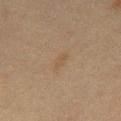Captured during whole-body skin photography for melanoma surveillance; the lesion was not biopsied.
A male patient, aged 43 to 47.
The lesion is located on the chest.
The lesion's longest dimension is about 3 mm.
A roughly 15 mm field-of-view crop from a total-body skin photograph.
The tile uses cross-polarized illumination.